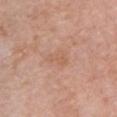This lesion was catalogued during total-body skin photography and was not selected for biopsy. From the chest. The tile uses white-light illumination. A 15 mm close-up extracted from a 3D total-body photography capture. About 3 mm across. A female patient, aged approximately 60.